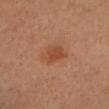<record>
<biopsy_status>not biopsied; imaged during a skin examination</biopsy_status>
<image>
  <source>total-body photography crop</source>
  <field_of_view_mm>15</field_of_view_mm>
</image>
<lesion_size>
  <long_diameter_mm_approx>3.5</long_diameter_mm_approx>
</lesion_size>
<site>head or neck</site>
<patient>
  <sex>female</sex>
  <age_approx>45</age_approx>
</patient>
<automated_metrics>
  <area_mm2_approx>7.5</area_mm2_approx>
  <eccentricity>0.7</eccentricity>
  <shape_asymmetry>0.15</shape_asymmetry>
  <cielab_L>49</cielab_L>
  <cielab_a>24</cielab_a>
  <cielab_b>35</cielab_b>
  <vs_skin_darker_L>7.0</vs_skin_darker_L>
  <vs_skin_contrast_norm>6.5</vs_skin_contrast_norm>
</automated_metrics>
</record>